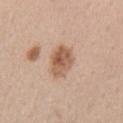biopsy status: catalogued during a skin exam; not biopsied
size: about 4 mm
lighting: white-light illumination
patient: female, aged 38 to 42
site: the left upper arm
image source: ~15 mm crop, total-body skin-cancer survey
automated metrics: an area of roughly 10 mm², an outline eccentricity of about 0.7 (0 = round, 1 = elongated), and a shape-asymmetry score of about 0.2 (0 = symmetric); about 12 CIELAB-L* units darker than the surrounding skin and a lesion-to-skin contrast of about 8 (normalized; higher = more distinct); internal color variation of about 6 on a 0–10 scale and radial color variation of about 2